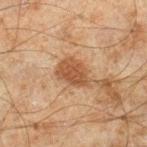The lesion was photographed on a routine skin check and not biopsied; there is no pathology result. A male patient, roughly 45 years of age. The lesion is on the leg. A roughly 15 mm field-of-view crop from a total-body skin photograph.